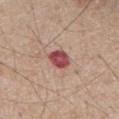This lesion was catalogued during total-body skin photography and was not selected for biopsy. Measured at roughly 3 mm in maximum diameter. On the chest. Cropped from a total-body skin-imaging series; the visible field is about 15 mm. The subject is a male in their mid- to late 60s. The tile uses white-light illumination.